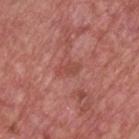Recorded during total-body skin imaging; not selected for excision or biopsy.